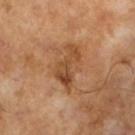Findings:
- follow-up: no biopsy performed (imaged during a skin exam)
- diameter: ~5 mm (longest diameter)
- subject: male, about 65 years old
- tile lighting: cross-polarized
- image-analysis metrics: a border-irregularity rating of about 6.5/10, a color-variation rating of about 6/10, and a peripheral color-asymmetry measure near 2
- image: ~15 mm tile from a whole-body skin photo